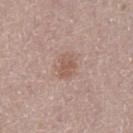workup: catalogued during a skin exam; not biopsied
body site: the left thigh
image: total-body-photography crop, ~15 mm field of view
patient: male, aged 73–77